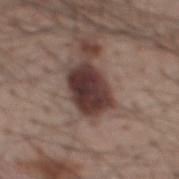The lesion was photographed on a routine skin check and not biopsied; there is no pathology result. Located on the mid back. A male subject, roughly 60 years of age. Cropped from a total-body skin-imaging series; the visible field is about 15 mm. Captured under white-light illumination. Longest diameter approximately 5 mm.